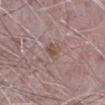Q: Was a biopsy performed?
A: total-body-photography surveillance lesion; no biopsy
Q: What did automated image analysis measure?
A: a lesion color around L≈53 a*≈17 b*≈23 in CIELAB, about 6 CIELAB-L* units darker than the surrounding skin, and a normalized border contrast of about 6; a classifier nevus-likeness of about 40/100 and a lesion-detection confidence of about 100/100
Q: Illumination type?
A: white-light illumination
Q: Where on the body is the lesion?
A: the abdomen
Q: What is the lesion's diameter?
A: ≈4 mm
Q: What are the patient's age and sex?
A: male, about 65 years old
Q: How was this image acquired?
A: ~15 mm crop, total-body skin-cancer survey